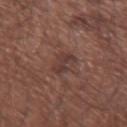Case summary:
• notes · total-body-photography surveillance lesion; no biopsy
• imaging modality · ~15 mm crop, total-body skin-cancer survey
• subject · male, roughly 65 years of age
• illumination · white-light
• location · the arm
• TBP lesion metrics · a lesion color around L≈37 a*≈19 b*≈21 in CIELAB, roughly 7 lightness units darker than nearby skin, and a normalized border contrast of about 6.5
• diameter · ~2.5 mm (longest diameter)